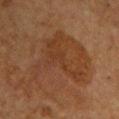workup=no biopsy performed (imaged during a skin exam); anatomic site=the chest; subject=female, in their 50s; imaging modality=~15 mm crop, total-body skin-cancer survey.